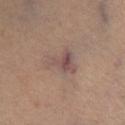Impression: No biopsy was performed on this lesion — it was imaged during a full skin examination and was not determined to be concerning. Clinical summary: A lesion tile, about 15 mm wide, cut from a 3D total-body photograph. A male patient, aged 48 to 52. The lesion is on the leg. The tile uses cross-polarized illumination. Automated tile analysis of the lesion measured roughly 7 lightness units darker than nearby skin and a lesion-to-skin contrast of about 6.5 (normalized; higher = more distinct). The analysis additionally found an automated nevus-likeness rating near 0 out of 100 and lesion-presence confidence of about 80/100. The recorded lesion diameter is about 4 mm.